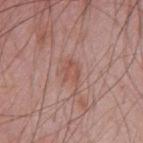Findings:
* workup: total-body-photography surveillance lesion; no biopsy